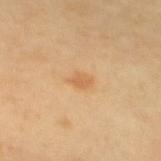Q: Was this lesion biopsied?
A: total-body-photography surveillance lesion; no biopsy
Q: How was this image acquired?
A: ~15 mm crop, total-body skin-cancer survey
Q: What is the lesion's diameter?
A: ~2.5 mm (longest diameter)
Q: What lighting was used for the tile?
A: cross-polarized illumination
Q: What is the anatomic site?
A: the upper back
Q: What did automated image analysis measure?
A: a mean CIELAB color near L≈64 a*≈22 b*≈41, roughly 8 lightness units darker than nearby skin, and a lesion-to-skin contrast of about 5.5 (normalized; higher = more distinct); border irregularity of about 2.5 on a 0–10 scale and internal color variation of about 1.5 on a 0–10 scale
Q: Patient demographics?
A: female, aged 58 to 62